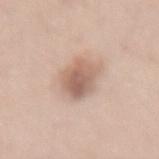follow-up = catalogued during a skin exam; not biopsied
anatomic site = the lower back
subject = female, roughly 45 years of age
image source = ~15 mm crop, total-body skin-cancer survey
size = ~4.5 mm (longest diameter)
illumination = white-light